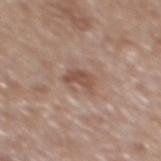| field | value |
|---|---|
| biopsy status | imaged on a skin check; not biopsied |
| subject | male, aged around 65 |
| size | about 3 mm |
| imaging modality | ~15 mm tile from a whole-body skin photo |
| illumination | white-light illumination |
| site | the mid back |